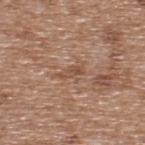biopsy status: imaged on a skin check; not biopsied | lesion diameter: about 3 mm | lighting: white-light | acquisition: ~15 mm tile from a whole-body skin photo | location: the upper back | image-analysis metrics: a lesion area of about 3 mm², a shape eccentricity near 0.9, and a shape-asymmetry score of about 0.25 (0 = symmetric); a classifier nevus-likeness of about 0/100 | patient: male, in their mid- to late 60s.